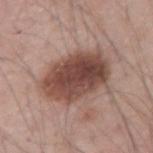Clinical impression:
The lesion was photographed on a routine skin check and not biopsied; there is no pathology result.
Acquisition and patient details:
The subject is a male aged approximately 45. The total-body-photography lesion software estimated a footprint of about 26 mm², a shape eccentricity near 0.75, and a shape-asymmetry score of about 0.15 (0 = symmetric). A 15 mm close-up tile from a total-body photography series done for melanoma screening. From the left upper arm. This is a white-light tile. Longest diameter approximately 7 mm.